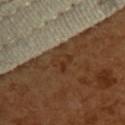* biopsy status · imaged on a skin check; not biopsied
* image · ~15 mm tile from a whole-body skin photo
* patient · female, aged 53 to 57
* location · the upper back
* lighting · cross-polarized illumination